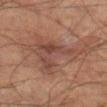Case summary:
• notes · no biopsy performed (imaged during a skin exam)
• imaging modality · 15 mm crop, total-body photography
• tile lighting · cross-polarized
• anatomic site · the left thigh
• automated lesion analysis · an average lesion color of about L≈39 a*≈18 b*≈23 (CIELAB), a lesion–skin lightness drop of about 7, and a lesion-to-skin contrast of about 6.5 (normalized; higher = more distinct)
• patient · male, aged approximately 65
• diameter · ≈8.5 mm